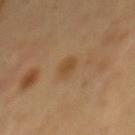Part of a total-body skin-imaging series; this lesion was reviewed on a skin check and was not flagged for biopsy. The recorded lesion diameter is about 2.5 mm. On the back. Captured under cross-polarized illumination. An algorithmic analysis of the crop reported a mean CIELAB color near L≈47 a*≈17 b*≈36, roughly 7 lightness units darker than nearby skin, and a lesion-to-skin contrast of about 6.5 (normalized; higher = more distinct). The analysis additionally found border irregularity of about 2.5 on a 0–10 scale, a color-variation rating of about 2/10, and peripheral color asymmetry of about 1. It also reported a classifier nevus-likeness of about 30/100 and a lesion-detection confidence of about 100/100. A male patient aged 48–52. Cropped from a total-body skin-imaging series; the visible field is about 15 mm.